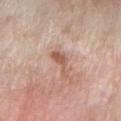• notes · no biopsy performed (imaged during a skin exam)
• image source · ~15 mm tile from a whole-body skin photo
• TBP lesion metrics · a lesion area of about 4 mm², an outline eccentricity of about 0.95 (0 = round, 1 = elongated), and a symmetry-axis asymmetry near 0.55; border irregularity of about 7 on a 0–10 scale, internal color variation of about 1.5 on a 0–10 scale, and peripheral color asymmetry of about 0.5; an automated nevus-likeness rating near 15 out of 100 and a detector confidence of about 100 out of 100 that the crop contains a lesion
• size · ≈4 mm
• subject · female, approximately 70 years of age
• illumination · white-light
• location · the right forearm